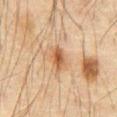biopsy status = total-body-photography surveillance lesion; no biopsy
patient = male, aged 63–67
automated lesion analysis = an area of roughly 5 mm² and a symmetry-axis asymmetry near 0.25; an average lesion color of about L≈46 a*≈18 b*≈31 (CIELAB), about 10 CIELAB-L* units darker than the surrounding skin, and a normalized border contrast of about 8; border irregularity of about 2.5 on a 0–10 scale and radial color variation of about 1
illumination = cross-polarized illumination
site = the abdomen
diameter = ≈3 mm
acquisition = ~15 mm tile from a whole-body skin photo